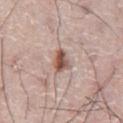| key | value |
|---|---|
| notes | no biopsy performed (imaged during a skin exam) |
| tile lighting | white-light illumination |
| image source | total-body-photography crop, ~15 mm field of view |
| lesion diameter | about 2.5 mm |
| automated lesion analysis | an area of roughly 4.5 mm²; an automated nevus-likeness rating near 90 out of 100 and lesion-presence confidence of about 100/100 |
| patient | male, about 75 years old |
| body site | the abdomen |